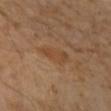This lesion was catalogued during total-body skin photography and was not selected for biopsy. On the right forearm. About 4 mm across. Cropped from a total-body skin-imaging series; the visible field is about 15 mm. A female subject aged 43–47. The tile uses cross-polarized illumination.